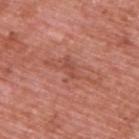follow-up: no biopsy performed (imaged during a skin exam); patient: male, roughly 70 years of age; location: the upper back; acquisition: total-body-photography crop, ~15 mm field of view; lesion size: about 6.5 mm.